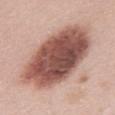Assessment: The lesion was tiled from a total-body skin photograph and was not biopsied. Acquisition and patient details: The tile uses white-light illumination. Located on the chest. This image is a 15 mm lesion crop taken from a total-body photograph. A female patient aged 48–52.